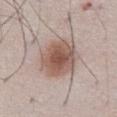Captured during whole-body skin photography for melanoma surveillance; the lesion was not biopsied.
A 15 mm close-up tile from a total-body photography series done for melanoma screening.
The tile uses white-light illumination.
A male subject, in their 50s.
From the abdomen.
Longest diameter approximately 6 mm.